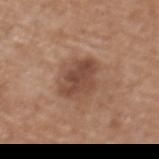| feature | finding |
|---|---|
| workup | imaged on a skin check; not biopsied |
| imaging modality | ~15 mm tile from a whole-body skin photo |
| subject | female, approximately 70 years of age |
| image-analysis metrics | a within-lesion color-variation index near 4/10 and radial color variation of about 1.5 |
| site | the upper back |
| lesion diameter | ≈4 mm |
| illumination | white-light |